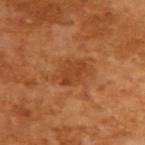Imaged during a routine full-body skin examination; the lesion was not biopsied and no histopathology is available. The lesion-visualizer software estimated a lesion area of about 8 mm², an eccentricity of roughly 0.7, and a shape-asymmetry score of about 0.3 (0 = symmetric). A close-up tile cropped from a whole-body skin photograph, about 15 mm across. The tile uses cross-polarized illumination. A male subject roughly 65 years of age. About 4 mm across.